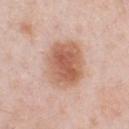Clinical impression:
This lesion was catalogued during total-body skin photography and was not selected for biopsy.